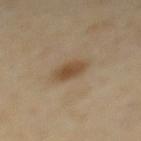follow-up=imaged on a skin check; not biopsied
size=≈3.5 mm
TBP lesion metrics=an eccentricity of roughly 0.7; a nevus-likeness score of about 90/100 and a detector confidence of about 100 out of 100 that the crop contains a lesion
anatomic site=the upper back
patient=female, aged around 45
illumination=cross-polarized
acquisition=total-body-photography crop, ~15 mm field of view